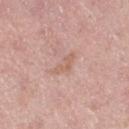{
  "biopsy_status": "not biopsied; imaged during a skin examination",
  "automated_metrics": {
    "area_mm2_approx": 4.0,
    "eccentricity": 0.9,
    "shape_asymmetry": 0.5,
    "cielab_L": 61,
    "cielab_a": 20,
    "cielab_b": 27,
    "vs_skin_darker_L": 7.0,
    "vs_skin_contrast_norm": 5.0,
    "border_irregularity_0_10": 5.0,
    "color_variation_0_10": 1.0,
    "peripheral_color_asymmetry": 0.0
  },
  "image": {
    "source": "total-body photography crop",
    "field_of_view_mm": 15
  },
  "lesion_size": {
    "long_diameter_mm_approx": 3.5
  },
  "lighting": "white-light",
  "patient": {
    "sex": "female",
    "age_approx": 50
  },
  "site": "right thigh"
}